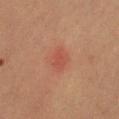<record>
  <biopsy_status>not biopsied; imaged during a skin examination</biopsy_status>
  <site>leg</site>
  <patient>
    <sex>female</sex>
    <age_approx>70</age_approx>
  </patient>
  <image>
    <source>total-body photography crop</source>
    <field_of_view_mm>15</field_of_view_mm>
  </image>
  <lighting>cross-polarized</lighting>
</record>